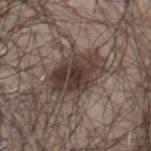subject: male, approximately 65 years of age
imaging modality: ~15 mm crop, total-body skin-cancer survey
diameter: about 6.5 mm
automated lesion analysis: an average lesion color of about L≈38 a*≈13 b*≈19 (CIELAB), roughly 12 lightness units darker than nearby skin, and a normalized lesion–skin contrast near 10; a nevus-likeness score of about 80/100
lighting: white-light illumination
anatomic site: the chest